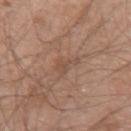Findings:
* workup — catalogued during a skin exam; not biopsied
* TBP lesion metrics — a lesion area of about 4 mm²
* location — the left upper arm
* size — ≈3 mm
* acquisition — 15 mm crop, total-body photography
* illumination — white-light
* subject — male, in their 20s